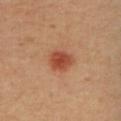Case summary:
• workup: catalogued during a skin exam; not biopsied
• patient: female, roughly 30 years of age
• site: the back
• lesion size: ≈3 mm
• tile lighting: cross-polarized
• image-analysis metrics: an average lesion color of about L≈48 a*≈29 b*≈35 (CIELAB), a lesion–skin lightness drop of about 12, and a lesion-to-skin contrast of about 9 (normalized; higher = more distinct)
• acquisition: ~15 mm crop, total-body skin-cancer survey